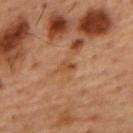| key | value |
|---|---|
| biopsy status | no biopsy performed (imaged during a skin exam) |
| image source | 15 mm crop, total-body photography |
| location | the back |
| patient | male, aged approximately 55 |
| illumination | cross-polarized illumination |